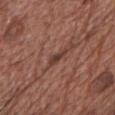workup = no biopsy performed (imaged during a skin exam)
lesion diameter = about 3 mm
imaging modality = ~15 mm tile from a whole-body skin photo
lighting = white-light
location = the chest
patient = female, about 75 years old